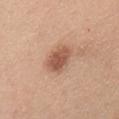Notes:
- biopsy status — no biopsy performed (imaged during a skin exam)
- lesion size — ≈4 mm
- image — 15 mm crop, total-body photography
- site — the chest
- image-analysis metrics — an area of roughly 8.5 mm²; a border-irregularity rating of about 2/10, a color-variation rating of about 3/10, and a peripheral color-asymmetry measure near 1; a nevus-likeness score of about 90/100 and lesion-presence confidence of about 100/100
- subject — female, aged around 45